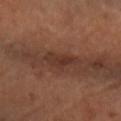Notes:
• tile lighting — cross-polarized illumination
• location — the right forearm
• lesion diameter — about 4 mm
• image source — 15 mm crop, total-body photography
• patient — female, aged 58 to 62
• automated metrics — a lesion area of about 6 mm² and an eccentricity of roughly 0.85; a mean CIELAB color near L≈34 a*≈21 b*≈26, roughly 8 lightness units darker than nearby skin, and a normalized border contrast of about 7; a border-irregularity index near 4/10, internal color variation of about 3.5 on a 0–10 scale, and a peripheral color-asymmetry measure near 1; an automated nevus-likeness rating near 0 out of 100 and a detector confidence of about 65 out of 100 that the crop contains a lesion